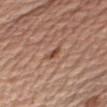Captured during whole-body skin photography for melanoma surveillance; the lesion was not biopsied.
On the right upper arm.
A region of skin cropped from a whole-body photographic capture, roughly 15 mm wide.
The tile uses white-light illumination.
Approximately 3 mm at its widest.
A male subject aged approximately 75.
Automated tile analysis of the lesion measured an area of roughly 2.5 mm², an outline eccentricity of about 0.95 (0 = round, 1 = elongated), and a symmetry-axis asymmetry near 0.45. It also reported about 10 CIELAB-L* units darker than the surrounding skin and a lesion-to-skin contrast of about 7.5 (normalized; higher = more distinct).